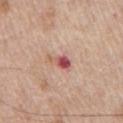Imaged during a routine full-body skin examination; the lesion was not biopsied and no histopathology is available. From the chest. A region of skin cropped from a whole-body photographic capture, roughly 15 mm wide. The subject is a male aged approximately 70.